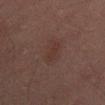Impression:
The lesion was tiled from a total-body skin photograph and was not biopsied.
Context:
A lesion tile, about 15 mm wide, cut from a 3D total-body photograph. The lesion is located on the mid back. The patient is a male about 45 years old.